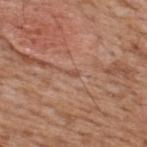Part of a total-body skin-imaging series; this lesion was reviewed on a skin check and was not flagged for biopsy.
This is a white-light tile.
A 15 mm crop from a total-body photograph taken for skin-cancer surveillance.
From the upper back.
A male patient, roughly 65 years of age.
The recorded lesion diameter is about 1.5 mm.